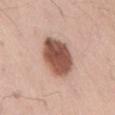Notes:
- workup — imaged on a skin check; not biopsied
- image source — total-body-photography crop, ~15 mm field of view
- lighting — white-light
- size — about 5 mm
- subject — male, aged 53–57
- body site — the lower back